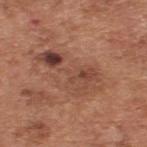Assessment:
The lesion was photographed on a routine skin check and not biopsied; there is no pathology result.
Context:
The patient is a male aged 63–67. Measured at roughly 7.5 mm in maximum diameter. A lesion tile, about 15 mm wide, cut from a 3D total-body photograph. The lesion is located on the upper back.